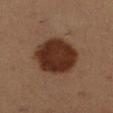Recorded during total-body skin imaging; not selected for excision or biopsy. Located on the leg. Measured at roughly 6 mm in maximum diameter. The tile uses cross-polarized illumination. A 15 mm crop from a total-body photograph taken for skin-cancer surveillance. A female subject, aged 23–27.